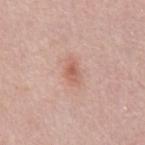This lesion was catalogued during total-body skin photography and was not selected for biopsy. Imaged with white-light lighting. From the chest. A male patient, aged 48–52. Automated tile analysis of the lesion measured a shape eccentricity near 0.85 and a shape-asymmetry score of about 0.2 (0 = symmetric). It also reported an average lesion color of about L≈60 a*≈23 b*≈28 (CIELAB) and a lesion-to-skin contrast of about 6.5 (normalized; higher = more distinct). The analysis additionally found an automated nevus-likeness rating near 30 out of 100. Cropped from a whole-body photographic skin survey; the tile spans about 15 mm.